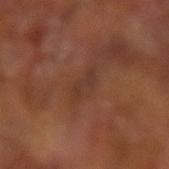This lesion was catalogued during total-body skin photography and was not selected for biopsy.
Approximately 3 mm at its widest.
Imaged with cross-polarized lighting.
The lesion is located on the left arm.
A region of skin cropped from a whole-body photographic capture, roughly 15 mm wide.
The subject is a male aged 63–67.